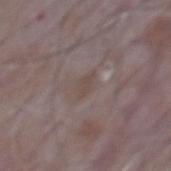Recorded during total-body skin imaging; not selected for excision or biopsy. On the mid back. The subject is a male roughly 65 years of age. A region of skin cropped from a whole-body photographic capture, roughly 15 mm wide.